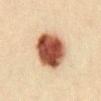Q: Is there a histopathology result?
A: catalogued during a skin exam; not biopsied
Q: Patient demographics?
A: female, aged approximately 40
Q: What is the imaging modality?
A: ~15 mm crop, total-body skin-cancer survey
Q: What is the lesion's diameter?
A: ~5.5 mm (longest diameter)
Q: Lesion location?
A: the front of the torso
Q: How was the tile lit?
A: cross-polarized